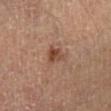Case summary:
– notes · total-body-photography surveillance lesion; no biopsy
– body site · the right lower leg
– TBP lesion metrics · a lesion area of about 4 mm², a shape eccentricity near 0.6, and a shape-asymmetry score of about 0.35 (0 = symmetric); a border-irregularity rating of about 3/10 and a within-lesion color-variation index near 4.5/10
– illumination · cross-polarized illumination
– patient · male, aged 63 to 67
– diameter · about 2.5 mm
– image source · total-body-photography crop, ~15 mm field of view